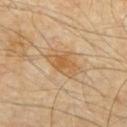The lesion was tiled from a total-body skin photograph and was not biopsied. Automated image analysis of the tile measured border irregularity of about 3.5 on a 0–10 scale, a color-variation rating of about 3.5/10, and a peripheral color-asymmetry measure near 1. And it measured a classifier nevus-likeness of about 65/100 and a detector confidence of about 100 out of 100 that the crop contains a lesion. The lesion is located on the chest. Captured under cross-polarized illumination. A 15 mm close-up tile from a total-body photography series done for melanoma screening. A male patient, roughly 60 years of age. Longest diameter approximately 4.5 mm.